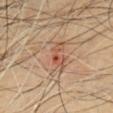Assessment:
Part of a total-body skin-imaging series; this lesion was reviewed on a skin check and was not flagged for biopsy.
Context:
Imaged with cross-polarized lighting. A 15 mm close-up extracted from a 3D total-body photography capture. Longest diameter approximately 6.5 mm. On the chest. A male subject, about 45 years old.